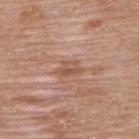Clinical impression: Captured during whole-body skin photography for melanoma surveillance; the lesion was not biopsied. Background: This is a white-light tile. A 15 mm close-up extracted from a 3D total-body photography capture. About 3 mm across. The lesion is on the upper back. The subject is a female approximately 60 years of age.